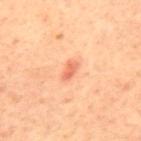Image and clinical context: The patient is a male aged 58 to 62. Located on the mid back. Cropped from a whole-body photographic skin survey; the tile spans about 15 mm.